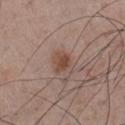Assessment: Captured during whole-body skin photography for melanoma surveillance; the lesion was not biopsied. Background: This image is a 15 mm lesion crop taken from a total-body photograph. From the chest. A male patient, about 55 years old.